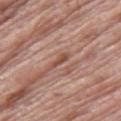biopsy status: catalogued during a skin exam; not biopsied | image source: ~15 mm tile from a whole-body skin photo | patient: male, aged 68–72 | diameter: ≈2.5 mm | illumination: white-light | body site: the leg.